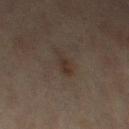follow-up=imaged on a skin check; not biopsied